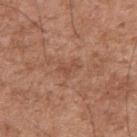Findings:
* lesion diameter · about 2.5 mm
* image · 15 mm crop, total-body photography
* location · the right upper arm
* TBP lesion metrics · a lesion color around L≈49 a*≈22 b*≈30 in CIELAB, about 6 CIELAB-L* units darker than the surrounding skin, and a normalized lesion–skin contrast near 5; a color-variation rating of about 0/10 and a peripheral color-asymmetry measure near 0
* subject · male, aged approximately 50